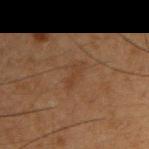Part of a total-body skin-imaging series; this lesion was reviewed on a skin check and was not flagged for biopsy.
The recorded lesion diameter is about 3 mm.
The subject is a male roughly 50 years of age.
Cropped from a whole-body photographic skin survey; the tile spans about 15 mm.
The lesion is on the right upper arm.
This is a cross-polarized tile.
Automated image analysis of the tile measured a symmetry-axis asymmetry near 0.25. And it measured a detector confidence of about 100 out of 100 that the crop contains a lesion.